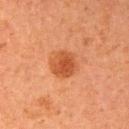Q: Is there a histopathology result?
A: total-body-photography surveillance lesion; no biopsy
Q: How large is the lesion?
A: ≈3.5 mm
Q: What is the anatomic site?
A: the right upper arm
Q: How was this image acquired?
A: total-body-photography crop, ~15 mm field of view
Q: How was the tile lit?
A: cross-polarized illumination
Q: Patient demographics?
A: female, aged 58–62